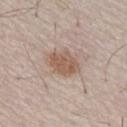Notes:
– biopsy status: imaged on a skin check; not biopsied
– lesion diameter: about 4 mm
– imaging modality: total-body-photography crop, ~15 mm field of view
– illumination: white-light
– anatomic site: the chest
– subject: male, aged approximately 65
– TBP lesion metrics: a border-irregularity index near 2.5/10 and internal color variation of about 2.5 on a 0–10 scale; a classifier nevus-likeness of about 65/100